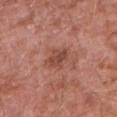Captured during whole-body skin photography for melanoma surveillance; the lesion was not biopsied.
The patient is a male roughly 60 years of age.
The lesion is located on the left upper arm.
An algorithmic analysis of the crop reported a nevus-likeness score of about 10/100 and a lesion-detection confidence of about 100/100.
Imaged with white-light lighting.
Approximately 3 mm at its widest.
A 15 mm close-up extracted from a 3D total-body photography capture.